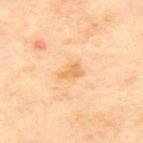The lesion was tiled from a total-body skin photograph and was not biopsied. This image is a 15 mm lesion crop taken from a total-body photograph. This is a cross-polarized tile. The subject is a female aged 58 to 62. The lesion is located on the upper back. The total-body-photography lesion software estimated an area of roughly 3.5 mm², a shape eccentricity near 0.75, and a symmetry-axis asymmetry near 0.45. The lesion's longest dimension is about 3 mm.